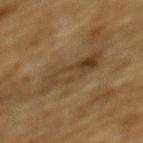{
  "biopsy_status": "not biopsied; imaged during a skin examination",
  "lighting": "cross-polarized",
  "site": "mid back",
  "image": {
    "source": "total-body photography crop",
    "field_of_view_mm": 15
  },
  "patient": {
    "sex": "male",
    "age_approx": "85+"
  }
}